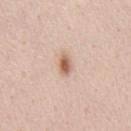Findings:
* notes · imaged on a skin check; not biopsied
* diameter · about 2.5 mm
* site · the chest
* imaging modality · 15 mm crop, total-body photography
* patient · male, about 45 years old
* automated lesion analysis · a mean CIELAB color near L≈62 a*≈20 b*≈30, roughly 14 lightness units darker than nearby skin, and a normalized lesion–skin contrast near 9; a border-irregularity index near 2/10, a color-variation rating of about 4.5/10, and peripheral color asymmetry of about 1.5; a lesion-detection confidence of about 100/100